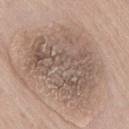Part of a total-body skin-imaging series; this lesion was reviewed on a skin check and was not flagged for biopsy. The subject is a male aged 73–77. On the lower back. Cropped from a whole-body photographic skin survey; the tile spans about 15 mm. Longest diameter approximately 9 mm. Automated image analysis of the tile measured a classifier nevus-likeness of about 0/100 and a lesion-detection confidence of about 100/100. Captured under white-light illumination.